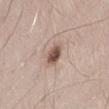Q: How was the tile lit?
A: white-light
Q: What kind of image is this?
A: ~15 mm tile from a whole-body skin photo
Q: Where on the body is the lesion?
A: the abdomen
Q: Patient demographics?
A: male, approximately 55 years of age
Q: What did automated image analysis measure?
A: a classifier nevus-likeness of about 95/100 and a lesion-detection confidence of about 100/100